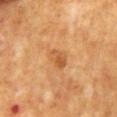Q: Was this lesion biopsied?
A: total-body-photography surveillance lesion; no biopsy
Q: Lesion size?
A: ~2.5 mm (longest diameter)
Q: What kind of image is this?
A: ~15 mm crop, total-body skin-cancer survey
Q: How was the tile lit?
A: cross-polarized illumination
Q: Who is the patient?
A: male, roughly 70 years of age
Q: What is the anatomic site?
A: the mid back